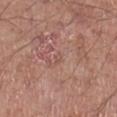<lesion>
<biopsy_status>not biopsied; imaged during a skin examination</biopsy_status>
<patient>
  <sex>male</sex>
  <age_approx>65</age_approx>
</patient>
<site>right lower leg</site>
<lesion_size>
  <long_diameter_mm_approx>1.0</long_diameter_mm_approx>
</lesion_size>
<image>
  <source>total-body photography crop</source>
  <field_of_view_mm>15</field_of_view_mm>
</image>
</lesion>